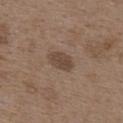The lesion was tiled from a total-body skin photograph and was not biopsied. Imaged with white-light lighting. This image is a 15 mm lesion crop taken from a total-body photograph. A female subject about 35 years old. Longest diameter approximately 3.5 mm. Automated tile analysis of the lesion measured an outline eccentricity of about 0.8 (0 = round, 1 = elongated). It also reported an automated nevus-likeness rating near 75 out of 100 and a lesion-detection confidence of about 100/100. Located on the upper back.